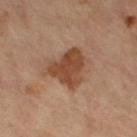Q: Is there a histopathology result?
A: catalogued during a skin exam; not biopsied
Q: What kind of image is this?
A: 15 mm crop, total-body photography
Q: Patient demographics?
A: female, aged around 65
Q: Where on the body is the lesion?
A: the left thigh